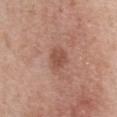Part of a total-body skin-imaging series; this lesion was reviewed on a skin check and was not flagged for biopsy. The lesion is on the chest. A female patient, aged around 45. An algorithmic analysis of the crop reported a lesion area of about 5 mm², an outline eccentricity of about 0.6 (0 = round, 1 = elongated), and two-axis asymmetry of about 0.25. The analysis additionally found a border-irregularity index near 2/10, a within-lesion color-variation index near 2.5/10, and a peripheral color-asymmetry measure near 1. And it measured an automated nevus-likeness rating near 10 out of 100 and a lesion-detection confidence of about 100/100. The lesion's longest dimension is about 2.5 mm. Imaged with white-light lighting. Cropped from a whole-body photographic skin survey; the tile spans about 15 mm.